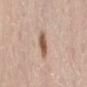| field | value |
|---|---|
| workup | no biopsy performed (imaged during a skin exam) |
| body site | the lower back |
| subject | female, aged approximately 65 |
| illumination | white-light illumination |
| image | 15 mm crop, total-body photography |